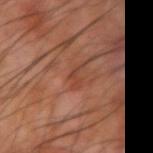The lesion was tiled from a total-body skin photograph and was not biopsied.
This image is a 15 mm lesion crop taken from a total-body photograph.
Imaged with cross-polarized lighting.
Located on the left forearm.
The subject is a male aged approximately 70.
The total-body-photography lesion software estimated a classifier nevus-likeness of about 0/100 and a detector confidence of about 100 out of 100 that the crop contains a lesion.